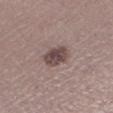The lesion was tiled from a total-body skin photograph and was not biopsied.
A female subject, aged around 40.
This is a white-light tile.
The lesion is located on the leg.
Approximately 3.5 mm at its widest.
A lesion tile, about 15 mm wide, cut from a 3D total-body photograph.